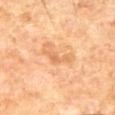Clinical impression:
Part of a total-body skin-imaging series; this lesion was reviewed on a skin check and was not flagged for biopsy.
Acquisition and patient details:
Longest diameter approximately 3 mm. This is a cross-polarized tile. A lesion tile, about 15 mm wide, cut from a 3D total-body photograph. A male patient, aged approximately 65. The lesion is located on the front of the torso.